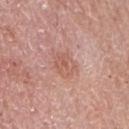Assessment:
This lesion was catalogued during total-body skin photography and was not selected for biopsy.
Background:
This image is a 15 mm lesion crop taken from a total-body photograph. The lesion is located on the right upper arm. The lesion-visualizer software estimated a footprint of about 6 mm², a shape eccentricity near 0.65, and a shape-asymmetry score of about 0.2 (0 = symmetric). And it measured a nevus-likeness score of about 0/100 and a lesion-detection confidence of about 100/100. A female patient, about 65 years old. Imaged with white-light lighting. The recorded lesion diameter is about 3 mm.